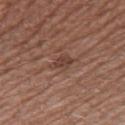notes = catalogued during a skin exam; not biopsied
diameter = ≈2.5 mm
image source = total-body-photography crop, ~15 mm field of view
body site = the right thigh
subject = male, aged approximately 65